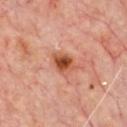– workup: total-body-photography surveillance lesion; no biopsy
– illumination: cross-polarized
– size: ~3 mm (longest diameter)
– subject: male, in their mid-60s
– image: total-body-photography crop, ~15 mm field of view
– location: the chest